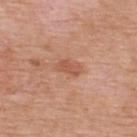<record>
  <biopsy_status>not biopsied; imaged during a skin examination</biopsy_status>
  <image>
    <source>total-body photography crop</source>
    <field_of_view_mm>15</field_of_view_mm>
  </image>
  <patient>
    <sex>male</sex>
    <age_approx>65</age_approx>
  </patient>
  <automated_metrics>
    <cielab_L>54</cielab_L>
    <cielab_a>26</cielab_a>
    <cielab_b>32</cielab_b>
    <vs_skin_contrast_norm>6.0</vs_skin_contrast_norm>
    <border_irregularity_0_10>2.5</border_irregularity_0_10>
    <color_variation_0_10>1.5</color_variation_0_10>
  </automated_metrics>
  <site>upper back</site>
  <lighting>white-light</lighting>
  <lesion_size>
    <long_diameter_mm_approx>2.5</long_diameter_mm_approx>
  </lesion_size>
</record>